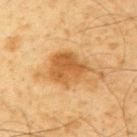Notes:
* biopsy status — total-body-photography surveillance lesion; no biopsy
* TBP lesion metrics — a lesion area of about 13 mm², a shape eccentricity near 0.55, and a symmetry-axis asymmetry near 0.3; a mean CIELAB color near L≈48 a*≈19 b*≈38, roughly 10 lightness units darker than nearby skin, and a normalized lesion–skin contrast near 8
* anatomic site — the arm
* tile lighting — cross-polarized
* diameter — ~5 mm (longest diameter)
* image — total-body-photography crop, ~15 mm field of view
* subject — male, aged 68 to 72